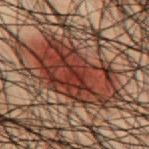{
  "site": "mid back",
  "patient": {
    "sex": "male",
    "age_approx": 50
  },
  "lighting": "cross-polarized",
  "automated_metrics": {
    "shape_asymmetry": 0.25,
    "cielab_L": 27,
    "cielab_a": 20,
    "cielab_b": 22,
    "vs_skin_darker_L": 10.0,
    "vs_skin_contrast_norm": 10.5
  },
  "image": {
    "source": "total-body photography crop",
    "field_of_view_mm": 15
  },
  "lesion_size": {
    "long_diameter_mm_approx": 9.0
  }
}